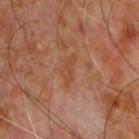{"biopsy_status": "not biopsied; imaged during a skin examination", "lighting": "cross-polarized", "image": {"source": "total-body photography crop", "field_of_view_mm": 15}, "site": "chest", "automated_metrics": {"eccentricity": 0.9, "shape_asymmetry": 0.45, "border_irregularity_0_10": 5.0, "peripheral_color_asymmetry": 0.0, "nevus_likeness_0_100": 0, "lesion_detection_confidence_0_100": 100}, "patient": {"sex": "male", "age_approx": 60}, "lesion_size": {"long_diameter_mm_approx": 3.0}}